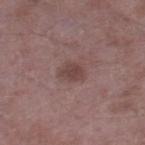image source: total-body-photography crop, ~15 mm field of view | patient: male, about 50 years old | anatomic site: the leg.